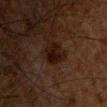Q: Is there a histopathology result?
A: imaged on a skin check; not biopsied
Q: How large is the lesion?
A: ≈3 mm
Q: How was the tile lit?
A: cross-polarized illumination
Q: How was this image acquired?
A: 15 mm crop, total-body photography
Q: What is the anatomic site?
A: the chest
Q: What are the patient's age and sex?
A: male, in their mid-60s